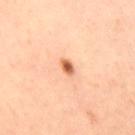Q: Was this lesion biopsied?
A: imaged on a skin check; not biopsied
Q: What kind of image is this?
A: ~15 mm crop, total-body skin-cancer survey
Q: What did automated image analysis measure?
A: a lesion color around L≈65 a*≈28 b*≈39 in CIELAB, about 17 CIELAB-L* units darker than the surrounding skin, and a normalized lesion–skin contrast near 10; a within-lesion color-variation index near 2.5/10 and peripheral color asymmetry of about 0.5; a classifier nevus-likeness of about 100/100
Q: How was the tile lit?
A: cross-polarized illumination
Q: Who is the patient?
A: female, aged around 40
Q: What is the anatomic site?
A: the right thigh